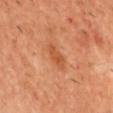Findings:
- notes — imaged on a skin check; not biopsied
- body site — the chest
- patient — male, aged approximately 40
- image — ~15 mm tile from a whole-body skin photo
- tile lighting — cross-polarized illumination
- lesion size — ~3 mm (longest diameter)